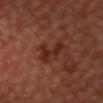Clinical summary: The subject is a male aged approximately 55. The lesion-visualizer software estimated a lesion color around L≈27 a*≈24 b*≈27 in CIELAB and roughly 8 lightness units darker than nearby skin. And it measured a border-irregularity rating of about 8/10, a color-variation rating of about 1/10, and a peripheral color-asymmetry measure near 0. Approximately 4 mm at its widest. Cropped from a total-body skin-imaging series; the visible field is about 15 mm. The lesion is located on the head or neck. Imaged with cross-polarized lighting.